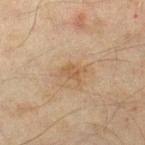The lesion was tiled from a total-body skin photograph and was not biopsied. The total-body-photography lesion software estimated a shape eccentricity near 0.75 and a shape-asymmetry score of about 0.4 (0 = symmetric). The analysis additionally found a peripheral color-asymmetry measure near 0. Imaged with cross-polarized lighting. A male patient aged 43–47. Located on the right lower leg. A 15 mm crop from a total-body photograph taken for skin-cancer surveillance.